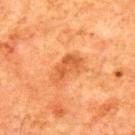Q: Where on the body is the lesion?
A: the upper back
Q: What kind of image is this?
A: 15 mm crop, total-body photography
Q: What are the patient's age and sex?
A: male, roughly 80 years of age
Q: How large is the lesion?
A: ≈4.5 mm
Q: What did automated image analysis measure?
A: an eccentricity of roughly 0.85 and two-axis asymmetry of about 0.25; a lesion-detection confidence of about 100/100
Q: How was the tile lit?
A: cross-polarized illumination